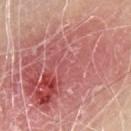| field | value |
|---|---|
| notes | imaged on a skin check; not biopsied |
| image source | ~15 mm tile from a whole-body skin photo |
| body site | the chest |
| subject | male, aged approximately 65 |